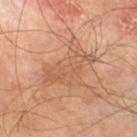Clinical summary: Cropped from a whole-body photographic skin survey; the tile spans about 15 mm. Automated image analysis of the tile measured a nevus-likeness score of about 0/100. A male subject aged 68–72. The lesion is located on the right thigh.